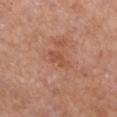- follow-up: no biopsy performed (imaged during a skin exam)
- location: the chest
- patient: female, about 60 years old
- tile lighting: white-light illumination
- lesion diameter: ~3.5 mm (longest diameter)
- image-analysis metrics: an outline eccentricity of about 0.9 (0 = round, 1 = elongated) and a symmetry-axis asymmetry near 0.25; a nevus-likeness score of about 0/100 and lesion-presence confidence of about 100/100
- acquisition: ~15 mm tile from a whole-body skin photo